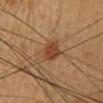Recorded during total-body skin imaging; not selected for excision or biopsy. This is a cross-polarized tile. The lesion is on the head or neck. A close-up tile cropped from a whole-body skin photograph, about 15 mm across. A female patient, aged 38 to 42. Longest diameter approximately 3 mm. Automated image analysis of the tile measured an area of roughly 5.5 mm², an eccentricity of roughly 0.75, and a shape-asymmetry score of about 0.2 (0 = symmetric). The software also gave about 8 CIELAB-L* units darker than the surrounding skin and a normalized lesion–skin contrast near 8. The software also gave a classifier nevus-likeness of about 85/100 and a detector confidence of about 100 out of 100 that the crop contains a lesion.